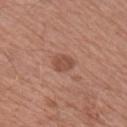| feature | finding |
|---|---|
| workup | no biopsy performed (imaged during a skin exam) |
| subject | male, aged around 65 |
| anatomic site | the right upper arm |
| imaging modality | total-body-photography crop, ~15 mm field of view |
| size | ~2.5 mm (longest diameter) |